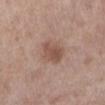notes — no biopsy performed (imaged during a skin exam); tile lighting — white-light illumination; size — ≈3.5 mm; body site — the left lower leg; image source — ~15 mm crop, total-body skin-cancer survey; patient — female, roughly 55 years of age.